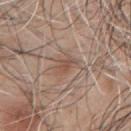The lesion was tiled from a total-body skin photograph and was not biopsied.
The lesion is located on the left upper arm.
A 15 mm close-up extracted from a 3D total-body photography capture.
The subject is a male aged 43 to 47.